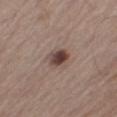biopsy status = catalogued during a skin exam; not biopsied | acquisition = 15 mm crop, total-body photography | site = the right thigh | patient = male, approximately 65 years of age.